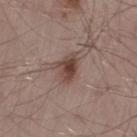Part of a total-body skin-imaging series; this lesion was reviewed on a skin check and was not flagged for biopsy. About 3.5 mm across. A roughly 15 mm field-of-view crop from a total-body skin photograph. A male subject approximately 55 years of age. The lesion-visualizer software estimated a footprint of about 6.5 mm², an eccentricity of roughly 0.7, and a shape-asymmetry score of about 0.25 (0 = symmetric). The analysis additionally found an automated nevus-likeness rating near 95 out of 100 and lesion-presence confidence of about 100/100. Imaged with white-light lighting. Located on the left thigh.